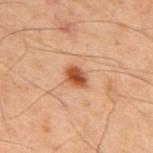| key | value |
|---|---|
| notes | no biopsy performed (imaged during a skin exam) |
| site | the back |
| tile lighting | cross-polarized |
| lesion diameter | about 2.5 mm |
| TBP lesion metrics | a lesion color around L≈40 a*≈21 b*≈31 in CIELAB, roughly 12 lightness units darker than nearby skin, and a lesion-to-skin contrast of about 10 (normalized; higher = more distinct); a color-variation rating of about 4/10; a classifier nevus-likeness of about 100/100 and a lesion-detection confidence of about 100/100 |
| patient | male, roughly 60 years of age |
| image source | ~15 mm tile from a whole-body skin photo |